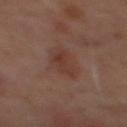biopsy status: imaged on a skin check; not biopsied | diameter: ≈5 mm | image: 15 mm crop, total-body photography | patient: male, aged 58 to 62 | illumination: cross-polarized | body site: the mid back | automated metrics: an outline eccentricity of about 0.9 (0 = round, 1 = elongated) and a shape-asymmetry score of about 0.35 (0 = symmetric); a mean CIELAB color near L≈33 a*≈19 b*≈22 and a normalized lesion–skin contrast near 6.5; an automated nevus-likeness rating near 75 out of 100 and a detector confidence of about 100 out of 100 that the crop contains a lesion.